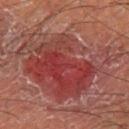Clinical impression: The lesion was photographed on a routine skin check and not biopsied; there is no pathology result. Clinical summary: This image is a 15 mm lesion crop taken from a total-body photograph. The patient is a male approximately 55 years of age. The tile uses cross-polarized illumination. The lesion is on the leg. Automated tile analysis of the lesion measured a footprint of about 60 mm², an outline eccentricity of about 0.7 (0 = round, 1 = elongated), and a shape-asymmetry score of about 0.4 (0 = symmetric). The software also gave roughly 8 lightness units darker than nearby skin and a lesion-to-skin contrast of about 7 (normalized; higher = more distinct).